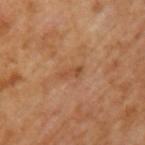Recorded during total-body skin imaging; not selected for excision or biopsy.
This image is a 15 mm lesion crop taken from a total-body photograph.
A male subject, in their mid- to late 60s.
The tile uses cross-polarized illumination.
Longest diameter approximately 3 mm.